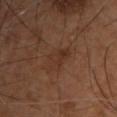Captured during whole-body skin photography for melanoma surveillance; the lesion was not biopsied. The patient is a male approximately 60 years of age. A region of skin cropped from a whole-body photographic capture, roughly 15 mm wide. Located on the chest.